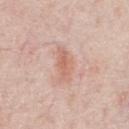Q: Was this lesion biopsied?
A: total-body-photography surveillance lesion; no biopsy